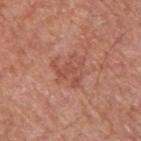Impression:
The lesion was tiled from a total-body skin photograph and was not biopsied.
Image and clinical context:
On the upper back. A male patient in their mid- to late 60s. An algorithmic analysis of the crop reported a border-irregularity rating of about 6.5/10, a color-variation rating of about 3/10, and a peripheral color-asymmetry measure near 1. The lesion's longest dimension is about 4 mm. This is a white-light tile. A 15 mm close-up extracted from a 3D total-body photography capture.